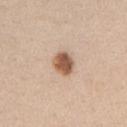Background:
The lesion is on the arm. This is a white-light tile. The recorded lesion diameter is about 3 mm. Automated image analysis of the tile measured a footprint of about 6.5 mm², an outline eccentricity of about 0.5 (0 = round, 1 = elongated), and two-axis asymmetry of about 0.15. And it measured border irregularity of about 1.5 on a 0–10 scale, a color-variation rating of about 4.5/10, and radial color variation of about 1.5. The subject is a female in their 40s. A 15 mm crop from a total-body photograph taken for skin-cancer surveillance.